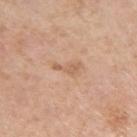Clinical impression:
Imaged during a routine full-body skin examination; the lesion was not biopsied and no histopathology is available.
Image and clinical context:
Automated image analysis of the tile measured an average lesion color of about L≈61 a*≈20 b*≈33 (CIELAB) and about 8 CIELAB-L* units darker than the surrounding skin. A 15 mm close-up extracted from a 3D total-body photography capture. About 3.5 mm across. The subject is a male aged approximately 65. Captured under white-light illumination. The lesion is located on the left upper arm.